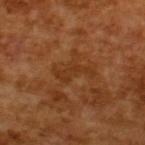Assessment: No biopsy was performed on this lesion — it was imaged during a full skin examination and was not determined to be concerning. Acquisition and patient details: Approximately 4 mm at its widest. A close-up tile cropped from a whole-body skin photograph, about 15 mm across. A male subject, aged approximately 65. Imaged with cross-polarized lighting.